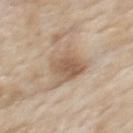{
  "biopsy_status": "not biopsied; imaged during a skin examination",
  "patient": {
    "sex": "male",
    "age_approx": "85+"
  },
  "lighting": "white-light",
  "site": "upper back",
  "lesion_size": {
    "long_diameter_mm_approx": 5.0
  },
  "image": {
    "source": "total-body photography crop",
    "field_of_view_mm": 15
  }
}